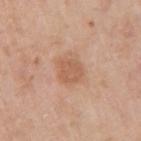Q: Is there a histopathology result?
A: total-body-photography surveillance lesion; no biopsy